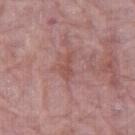<lesion>
  <biopsy_status>not biopsied; imaged during a skin examination</biopsy_status>
  <patient>
    <sex>female</sex>
    <age_approx>65</age_approx>
  </patient>
  <image>
    <source>total-body photography crop</source>
    <field_of_view_mm>15</field_of_view_mm>
  </image>
  <lesion_size>
    <long_diameter_mm_approx>3.0</long_diameter_mm_approx>
  </lesion_size>
  <site>leg</site>
</lesion>